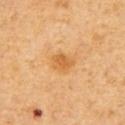Part of a total-body skin-imaging series; this lesion was reviewed on a skin check and was not flagged for biopsy.